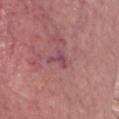lighting: white-light
site: head or neck
lesion_size:
  long_diameter_mm_approx: 3.0
image:
  source: total-body photography crop
  field_of_view_mm: 15
patient:
  sex: male
  age_approx: 65